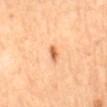{"biopsy_status": "not biopsied; imaged during a skin examination", "patient": {"sex": "female", "age_approx": 65}, "lesion_size": {"long_diameter_mm_approx": 2.5}, "image": {"source": "total-body photography crop", "field_of_view_mm": 15}, "automated_metrics": {"border_irregularity_0_10": 3.0, "peripheral_color_asymmetry": 0.0}, "site": "mid back", "lighting": "cross-polarized"}